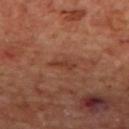Background:
The recorded lesion diameter is about 2.5 mm. Captured under cross-polarized illumination. Cropped from a total-body skin-imaging series; the visible field is about 15 mm. On the mid back. The patient is a male aged around 70.